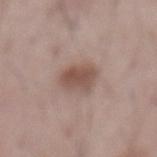Image and clinical context: A 15 mm close-up extracted from a 3D total-body photography capture. The lesion is located on the front of the torso. A male patient, in their mid- to late 50s.